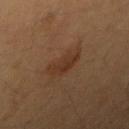A 15 mm crop from a total-body photograph taken for skin-cancer surveillance.
On the arm.
A male subject, in their 40s.
The total-body-photography lesion software estimated a lesion area of about 7 mm², a shape eccentricity near 0.85, and a shape-asymmetry score of about 0.35 (0 = symmetric). And it measured a mean CIELAB color near L≈29 a*≈17 b*≈26, roughly 6 lightness units darker than nearby skin, and a normalized lesion–skin contrast near 7.